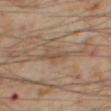Impression:
Imaged during a routine full-body skin examination; the lesion was not biopsied and no histopathology is available.
Background:
An algorithmic analysis of the crop reported an eccentricity of roughly 0.9 and two-axis asymmetry of about 0.4. A male patient, in their mid-50s. Located on the right thigh. A close-up tile cropped from a whole-body skin photograph, about 15 mm across. This is a cross-polarized tile. The lesion's longest dimension is about 3 mm.